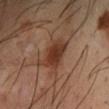Image and clinical context: On the left forearm. The lesion's longest dimension is about 4.5 mm. Captured under cross-polarized illumination. Automated tile analysis of the lesion measured a lesion color around L≈36 a*≈21 b*≈28 in CIELAB and a lesion-to-skin contrast of about 9 (normalized; higher = more distinct). The software also gave border irregularity of about 3.5 on a 0–10 scale and a color-variation rating of about 3.5/10. The software also gave a lesion-detection confidence of about 100/100. A male subject aged around 40. A 15 mm close-up extracted from a 3D total-body photography capture.